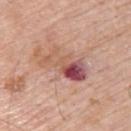Captured during whole-body skin photography for melanoma surveillance; the lesion was not biopsied. The patient is a male approximately 60 years of age. A 15 mm crop from a total-body photograph taken for skin-cancer surveillance. Located on the upper back. The lesion's longest dimension is about 5.5 mm.